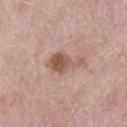{
  "biopsy_status": "not biopsied; imaged during a skin examination",
  "image": {
    "source": "total-body photography crop",
    "field_of_view_mm": 15
  },
  "lesion_size": {
    "long_diameter_mm_approx": 4.5
  },
  "patient": {
    "sex": "female",
    "age_approx": 75
  },
  "site": "right lower leg",
  "lighting": "white-light"
}